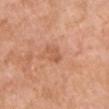<lesion>
<biopsy_status>not biopsied; imaged during a skin examination</biopsy_status>
<patient>
  <sex>female</sex>
  <age_approx>60</age_approx>
</patient>
<automated_metrics>
  <eccentricity>0.85</eccentricity>
  <shape_asymmetry>0.4</shape_asymmetry>
  <cielab_L>57</cielab_L>
  <cielab_a>26</cielab_a>
  <cielab_b>35</cielab_b>
  <vs_skin_darker_L>8.0</vs_skin_darker_L>
  <vs_skin_contrast_norm>5.5</vs_skin_contrast_norm>
  <border_irregularity_0_10>5.0</border_irregularity_0_10>
  <color_variation_0_10>0.0</color_variation_0_10>
  <peripheral_color_asymmetry>0.0</peripheral_color_asymmetry>
</automated_metrics>
<image>
  <source>total-body photography crop</source>
  <field_of_view_mm>15</field_of_view_mm>
</image>
<lesion_size>
  <long_diameter_mm_approx>2.5</long_diameter_mm_approx>
</lesion_size>
<site>chest</site>
<lighting>white-light</lighting>
</lesion>